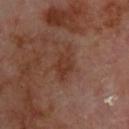| key | value |
|---|---|
| workup | imaged on a skin check; not biopsied |
| location | the upper back |
| size | ~4 mm (longest diameter) |
| patient | male, about 70 years old |
| imaging modality | ~15 mm tile from a whole-body skin photo |
| lighting | cross-polarized illumination |
| automated metrics | a footprint of about 6.5 mm² and a shape eccentricity near 0.8; a nevus-likeness score of about 10/100 and a detector confidence of about 100 out of 100 that the crop contains a lesion |